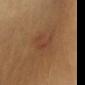Q: Was this lesion biopsied?
A: imaged on a skin check; not biopsied